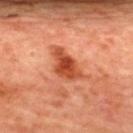The total-body-photography lesion software estimated an eccentricity of roughly 0.8 and a symmetry-axis asymmetry near 0.3. The analysis additionally found an average lesion color of about L≈50 a*≈33 b*≈39 (CIELAB), about 13 CIELAB-L* units darker than the surrounding skin, and a normalized border contrast of about 9. The analysis additionally found a detector confidence of about 100 out of 100 that the crop contains a lesion.
Located on the upper back.
Cropped from a total-body skin-imaging series; the visible field is about 15 mm.
A female patient, in their mid-40s.